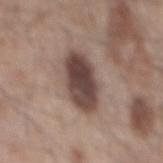Located on the back. Cropped from a whole-body photographic skin survey; the tile spans about 15 mm. A male subject, in their mid-50s.